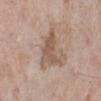patient = female, roughly 75 years of age
location = the left lower leg
acquisition = 15 mm crop, total-body photography